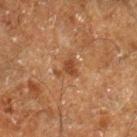Clinical impression: No biopsy was performed on this lesion — it was imaged during a full skin examination and was not determined to be concerning. Background: Cropped from a total-body skin-imaging series; the visible field is about 15 mm. Measured at roughly 3 mm in maximum diameter. The tile uses cross-polarized illumination. The lesion-visualizer software estimated a classifier nevus-likeness of about 5/100 and a detector confidence of about 100 out of 100 that the crop contains a lesion. A male patient, approximately 60 years of age. Located on the leg.